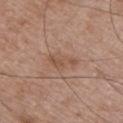Q: Was a biopsy performed?
A: no biopsy performed (imaged during a skin exam)
Q: What is the imaging modality?
A: ~15 mm crop, total-body skin-cancer survey
Q: What did automated image analysis measure?
A: a lesion area of about 5.5 mm² and a shape-asymmetry score of about 0.3 (0 = symmetric); roughly 7 lightness units darker than nearby skin and a normalized lesion–skin contrast near 5.5; a classifier nevus-likeness of about 0/100
Q: Where on the body is the lesion?
A: the chest
Q: Who is the patient?
A: male, aged approximately 50
Q: What lighting was used for the tile?
A: white-light